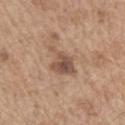| field | value |
|---|---|
| workup | imaged on a skin check; not biopsied |
| acquisition | ~15 mm tile from a whole-body skin photo |
| lesion size | ≈4.5 mm |
| illumination | white-light illumination |
| subject | male, roughly 70 years of age |
| site | the left upper arm |